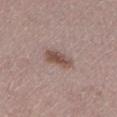biopsy status: total-body-photography surveillance lesion; no biopsy | imaging modality: ~15 mm tile from a whole-body skin photo | site: the leg | automated lesion analysis: an average lesion color of about L≈50 a*≈17 b*≈23 (CIELAB), about 11 CIELAB-L* units darker than the surrounding skin, and a lesion-to-skin contrast of about 8 (normalized; higher = more distinct); a border-irregularity rating of about 2.5/10, internal color variation of about 3 on a 0–10 scale, and peripheral color asymmetry of about 1; a classifier nevus-likeness of about 85/100 | diameter: ≈4 mm | lighting: white-light illumination | subject: male, aged approximately 70.